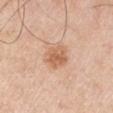Case summary:
• follow-up · catalogued during a skin exam; not biopsied
• acquisition · 15 mm crop, total-body photography
• size · ≈3.5 mm
• subject · male, aged 63 to 67
• image-analysis metrics · a lesion area of about 7.5 mm², a shape eccentricity near 0.5, and a symmetry-axis asymmetry near 0.2; an average lesion color of about L≈62 a*≈22 b*≈34 (CIELAB) and about 10 CIELAB-L* units darker than the surrounding skin; a border-irregularity rating of about 2/10, a color-variation rating of about 3.5/10, and a peripheral color-asymmetry measure near 1; a nevus-likeness score of about 70/100 and a lesion-detection confidence of about 100/100
• anatomic site · the right upper arm
• illumination · white-light illumination